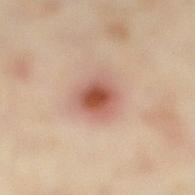Assessment: Imaged during a routine full-body skin examination; the lesion was not biopsied and no histopathology is available. Acquisition and patient details: From the right lower leg. The total-body-photography lesion software estimated roughly 13 lightness units darker than nearby skin and a lesion-to-skin contrast of about 8.5 (normalized; higher = more distinct). The analysis additionally found a border-irregularity rating of about 2/10, a within-lesion color-variation index near 7/10, and radial color variation of about 1.5. It also reported lesion-presence confidence of about 100/100. A female patient, aged 33 to 37. The tile uses cross-polarized illumination. Measured at roughly 4 mm in maximum diameter. Cropped from a total-body skin-imaging series; the visible field is about 15 mm.